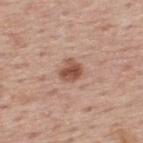<case>
<biopsy_status>not biopsied; imaged during a skin examination</biopsy_status>
<patient>
  <sex>male</sex>
  <age_approx>75</age_approx>
</patient>
<site>back</site>
<lesion_size>
  <long_diameter_mm_approx>2.5</long_diameter_mm_approx>
</lesion_size>
<image>
  <source>total-body photography crop</source>
  <field_of_view_mm>15</field_of_view_mm>
</image>
<lighting>white-light</lighting>
<automated_metrics>
  <cielab_L>52</cielab_L>
  <cielab_a>22</cielab_a>
  <cielab_b>28</cielab_b>
  <vs_skin_darker_L>13.0</vs_skin_darker_L>
  <border_irregularity_0_10>2.0</border_irregularity_0_10>
  <color_variation_0_10>4.0</color_variation_0_10>
</automated_metrics>
</case>